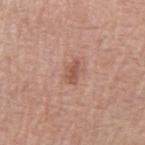Captured during whole-body skin photography for melanoma surveillance; the lesion was not biopsied. On the arm. A 15 mm close-up extracted from a 3D total-body photography capture. The subject is a female aged 53 to 57. This is a white-light tile. The recorded lesion diameter is about 3 mm. The lesion-visualizer software estimated an area of roughly 4 mm², an outline eccentricity of about 0.8 (0 = round, 1 = elongated), and two-axis asymmetry of about 0.25. The software also gave internal color variation of about 2 on a 0–10 scale and a peripheral color-asymmetry measure near 0.5.